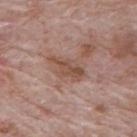Imaged during a routine full-body skin examination; the lesion was not biopsied and no histopathology is available. A male subject, aged approximately 60. A region of skin cropped from a whole-body photographic capture, roughly 15 mm wide. The lesion is located on the back. Automated tile analysis of the lesion measured a mean CIELAB color near L≈50 a*≈19 b*≈26, about 9 CIELAB-L* units darker than the surrounding skin, and a normalized lesion–skin contrast near 7. It also reported border irregularity of about 4 on a 0–10 scale, a within-lesion color-variation index near 3.5/10, and a peripheral color-asymmetry measure near 1. And it measured an automated nevus-likeness rating near 0 out of 100 and a lesion-detection confidence of about 100/100. This is a white-light tile. The recorded lesion diameter is about 4 mm.